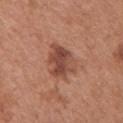Assessment:
The lesion was tiled from a total-body skin photograph and was not biopsied.
Background:
Imaged with white-light lighting. A 15 mm crop from a total-body photograph taken for skin-cancer surveillance. The subject is a female approximately 65 years of age. From the chest. About 4.5 mm across.